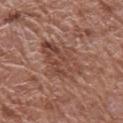Impression:
The lesion was photographed on a routine skin check and not biopsied; there is no pathology result.
Context:
A lesion tile, about 15 mm wide, cut from a 3D total-body photograph. Automated image analysis of the tile measured a footprint of about 19 mm², an eccentricity of roughly 0.8, and a shape-asymmetry score of about 0.25 (0 = symmetric). The analysis additionally found an average lesion color of about L≈46 a*≈22 b*≈27 (CIELAB). It also reported a border-irregularity rating of about 4/10, a color-variation rating of about 5.5/10, and radial color variation of about 2. The analysis additionally found an automated nevus-likeness rating near 0 out of 100. Located on the right upper arm. A female subject, roughly 80 years of age. Measured at roughly 7 mm in maximum diameter.